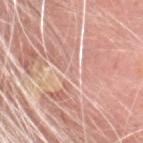lesion size: ~1 mm (longest diameter)
subject: male, about 80 years old
body site: the head or neck
TBP lesion metrics: an average lesion color of about L≈67 a*≈21 b*≈25 (CIELAB), about 5 CIELAB-L* units darker than the surrounding skin, and a normalized border contrast of about 3; a border-irregularity index near 4.5/10, a within-lesion color-variation index near 0/10, and a peripheral color-asymmetry measure near 0
acquisition: ~15 mm tile from a whole-body skin photo
pathology: an actinic keratosis (borderline)